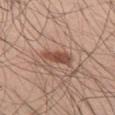{"lighting": "white-light", "lesion_size": {"long_diameter_mm_approx": 4.0}, "patient": {"sex": "male", "age_approx": 55}, "image": {"source": "total-body photography crop", "field_of_view_mm": 15}, "site": "left thigh"}